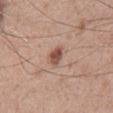• biopsy status — imaged on a skin check; not biopsied
• tile lighting — white-light
• site — the back
• automated lesion analysis — a footprint of about 4 mm², an outline eccentricity of about 0.8 (0 = round, 1 = elongated), and a symmetry-axis asymmetry near 0.25; a mean CIELAB color near L≈51 a*≈20 b*≈27 and about 13 CIELAB-L* units darker than the surrounding skin; a border-irregularity index near 2.5/10 and a peripheral color-asymmetry measure near 1
• image source — 15 mm crop, total-body photography
• subject — male, aged 48 to 52